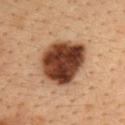anatomic site: the upper back
tile lighting: cross-polarized illumination
subject: male, aged 33–37
image: 15 mm crop, total-body photography
histopathologic diagnosis: a dysplastic (Clark) nevus